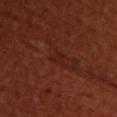* notes — no biopsy performed (imaged during a skin exam)
* subject — female, roughly 50 years of age
* imaging modality — total-body-photography crop, ~15 mm field of view